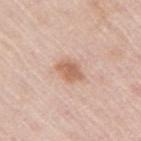follow-up: no biopsy performed (imaged during a skin exam) | image: 15 mm crop, total-body photography | body site: the right upper arm | lesion size: about 3 mm | illumination: white-light | patient: female, aged approximately 65.